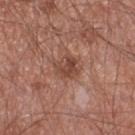This lesion was catalogued during total-body skin photography and was not selected for biopsy. Measured at roughly 3 mm in maximum diameter. A region of skin cropped from a whole-body photographic capture, roughly 15 mm wide. Captured under white-light illumination. The lesion is located on the left thigh. Automated image analysis of the tile measured a lesion-detection confidence of about 100/100. A male subject, approximately 55 years of age.